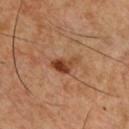The lesion was tiled from a total-body skin photograph and was not biopsied. The lesion's longest dimension is about 3.5 mm. Cropped from a whole-body photographic skin survey; the tile spans about 15 mm. Imaged with cross-polarized lighting. Automated image analysis of the tile measured a lesion color around L≈42 a*≈24 b*≈34 in CIELAB, roughly 12 lightness units darker than nearby skin, and a lesion-to-skin contrast of about 9.5 (normalized; higher = more distinct). And it measured border irregularity of about 4 on a 0–10 scale, a color-variation rating of about 6/10, and peripheral color asymmetry of about 2. The lesion is located on the chest. The patient is a male aged 48 to 52.